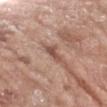Q: Is there a histopathology result?
A: total-body-photography surveillance lesion; no biopsy
Q: What did automated image analysis measure?
A: a lesion color around L≈51 a*≈21 b*≈26 in CIELAB and a normalized border contrast of about 7.5
Q: What are the patient's age and sex?
A: female, aged approximately 75
Q: Where on the body is the lesion?
A: the left forearm
Q: Illumination type?
A: white-light illumination
Q: What kind of image is this?
A: total-body-photography crop, ~15 mm field of view
Q: How large is the lesion?
A: ~3 mm (longest diameter)